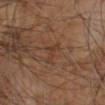  biopsy_status: not biopsied; imaged during a skin examination
  patient:
    sex: male
    age_approx: 60
  site: arm
  automated_metrics:
    eccentricity: 0.9
    shape_asymmetry: 0.5
  image:
    source: total-body photography crop
    field_of_view_mm: 15
  lesion_size:
    long_diameter_mm_approx: 3.0
  lighting: cross-polarized